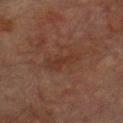notes: total-body-photography surveillance lesion; no biopsy.